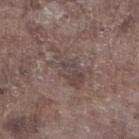Imaged during a routine full-body skin examination; the lesion was not biopsied and no histopathology is available. The tile uses white-light illumination. A 15 mm close-up tile from a total-body photography series done for melanoma screening. The lesion is located on the right lower leg. The patient is a male in their mid- to late 70s. An algorithmic analysis of the crop reported an average lesion color of about L≈43 a*≈14 b*≈18 (CIELAB) and roughly 7 lightness units darker than nearby skin. It also reported internal color variation of about 3 on a 0–10 scale and a peripheral color-asymmetry measure near 0.5. And it measured a nevus-likeness score of about 0/100 and a lesion-detection confidence of about 55/100.